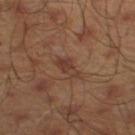Captured during whole-body skin photography for melanoma surveillance; the lesion was not biopsied. The subject is a male aged approximately 45. A lesion tile, about 15 mm wide, cut from a 3D total-body photograph. Located on the left lower leg. The tile uses cross-polarized illumination. Automated image analysis of the tile measured a mean CIELAB color near L≈37 a*≈19 b*≈26, roughly 7 lightness units darker than nearby skin, and a normalized lesion–skin contrast near 6.5. The software also gave a border-irregularity rating of about 3.5/10, a color-variation rating of about 2/10, and a peripheral color-asymmetry measure near 0.5. The analysis additionally found a classifier nevus-likeness of about 5/100 and a detector confidence of about 100 out of 100 that the crop contains a lesion.